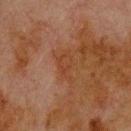Recorded during total-body skin imaging; not selected for excision or biopsy. On the upper back. The patient is a male approximately 80 years of age. A 15 mm close-up tile from a total-body photography series done for melanoma screening. Imaged with cross-polarized lighting.